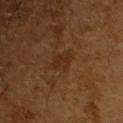Assessment: Recorded during total-body skin imaging; not selected for excision or biopsy. Acquisition and patient details: The lesion is located on the right upper arm. The lesion's longest dimension is about 3 mm. A 15 mm close-up tile from a total-body photography series done for melanoma screening. Automated tile analysis of the lesion measured a nevus-likeness score of about 0/100 and lesion-presence confidence of about 100/100. The subject is a male aged 63 to 67.